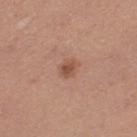Imaged during a routine full-body skin examination; the lesion was not biopsied and no histopathology is available.
On the left thigh.
Imaged with white-light lighting.
Cropped from a total-body skin-imaging series; the visible field is about 15 mm.
Automated image analysis of the tile measured a lesion color around L≈52 a*≈22 b*≈29 in CIELAB, about 9 CIELAB-L* units darker than the surrounding skin, and a lesion-to-skin contrast of about 6.5 (normalized; higher = more distinct).
A female subject, approximately 35 years of age.
The recorded lesion diameter is about 2.5 mm.